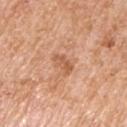Findings:
* workup — no biopsy performed (imaged during a skin exam)
* subject — male, approximately 60 years of age
* illumination — white-light
* body site — the left upper arm
* diameter — about 2.5 mm
* image-analysis metrics — a mean CIELAB color near L≈59 a*≈24 b*≈35 and about 9 CIELAB-L* units darker than the surrounding skin; a color-variation rating of about 2/10 and radial color variation of about 1; a nevus-likeness score of about 0/100 and lesion-presence confidence of about 100/100
* image source — ~15 mm crop, total-body skin-cancer survey